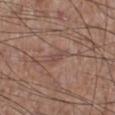Impression: No biopsy was performed on this lesion — it was imaged during a full skin examination and was not determined to be concerning. Clinical summary: Captured under white-light illumination. Approximately 2.5 mm at its widest. A male patient, in their mid-60s. This image is a 15 mm lesion crop taken from a total-body photograph. An algorithmic analysis of the crop reported an automated nevus-likeness rating near 0 out of 100. From the right lower leg.